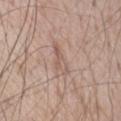Findings:
* biopsy status: catalogued during a skin exam; not biopsied
* subject: male, aged 53–57
* tile lighting: white-light
* image: ~15 mm crop, total-body skin-cancer survey